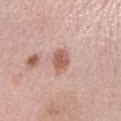Part of a total-body skin-imaging series; this lesion was reviewed on a skin check and was not flagged for biopsy.
A female patient, aged 63 to 67.
An algorithmic analysis of the crop reported a mean CIELAB color near L≈59 a*≈23 b*≈27, about 12 CIELAB-L* units darker than the surrounding skin, and a lesion-to-skin contrast of about 8 (normalized; higher = more distinct). And it measured a border-irregularity rating of about 2/10 and a within-lesion color-variation index near 4/10.
Cropped from a whole-body photographic skin survey; the tile spans about 15 mm.
The lesion is on the left lower leg.